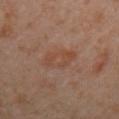Impression:
No biopsy was performed on this lesion — it was imaged during a full skin examination and was not determined to be concerning.
Image and clinical context:
An algorithmic analysis of the crop reported an area of roughly 8.5 mm², an eccentricity of roughly 0.75, and two-axis asymmetry of about 0.2. The software also gave an average lesion color of about L≈44 a*≈19 b*≈28 (CIELAB), about 5 CIELAB-L* units darker than the surrounding skin, and a lesion-to-skin contrast of about 5 (normalized; higher = more distinct). The software also gave a border-irregularity rating of about 2.5/10, a within-lesion color-variation index near 4/10, and peripheral color asymmetry of about 1.5. Cropped from a whole-body photographic skin survey; the tile spans about 15 mm. Approximately 4 mm at its widest. The tile uses cross-polarized illumination. A female subject, aged 48 to 52. On the right upper arm.